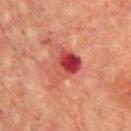workup = catalogued during a skin exam; not biopsied.